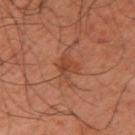Imaged during a routine full-body skin examination; the lesion was not biopsied and no histopathology is available. On the right upper arm. A male subject aged approximately 40. This image is a 15 mm lesion crop taken from a total-body photograph.